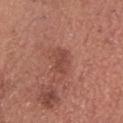Part of a total-body skin-imaging series; this lesion was reviewed on a skin check and was not flagged for biopsy.
A close-up tile cropped from a whole-body skin photograph, about 15 mm across.
From the head or neck.
A male subject approximately 75 years of age.
The total-body-photography lesion software estimated a footprint of about 4.5 mm², an eccentricity of roughly 0.8, and a symmetry-axis asymmetry near 0.3. It also reported a mean CIELAB color near L≈46 a*≈25 b*≈27, a lesion–skin lightness drop of about 8, and a lesion-to-skin contrast of about 6 (normalized; higher = more distinct).
About 3 mm across.